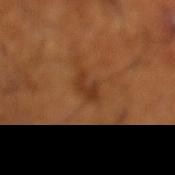* biopsy status — imaged on a skin check; not biopsied
* anatomic site — the left lower leg
* image — ~15 mm tile from a whole-body skin photo
* patient — male, aged 63 to 67
* illumination — cross-polarized illumination
* size — about 3 mm
* automated metrics — an area of roughly 4.5 mm² and an eccentricity of roughly 0.75; a border-irregularity index near 3.5/10, internal color variation of about 2 on a 0–10 scale, and peripheral color asymmetry of about 0.5; a nevus-likeness score of about 0/100